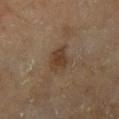No biopsy was performed on this lesion — it was imaged during a full skin examination and was not determined to be concerning.
Measured at roughly 3 mm in maximum diameter.
On the right lower leg.
The subject is a male approximately 65 years of age.
This image is a 15 mm lesion crop taken from a total-body photograph.